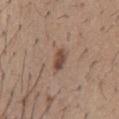Imaged during a routine full-body skin examination; the lesion was not biopsied and no histopathology is available. The lesion is on the chest. A male patient, aged approximately 60. The tile uses white-light illumination. Automated tile analysis of the lesion measured border irregularity of about 2 on a 0–10 scale, internal color variation of about 2 on a 0–10 scale, and peripheral color asymmetry of about 0.5. And it measured a classifier nevus-likeness of about 90/100 and lesion-presence confidence of about 100/100. This image is a 15 mm lesion crop taken from a total-body photograph.